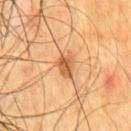Recorded during total-body skin imaging; not selected for excision or biopsy.
About 3 mm across.
On the chest.
This is a cross-polarized tile.
An algorithmic analysis of the crop reported an area of roughly 5 mm², an eccentricity of roughly 0.75, and a shape-asymmetry score of about 0.25 (0 = symmetric). The software also gave a border-irregularity index near 2.5/10.
The subject is a male in their mid-50s.
A 15 mm close-up extracted from a 3D total-body photography capture.